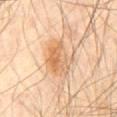Captured during whole-body skin photography for melanoma surveillance; the lesion was not biopsied. Measured at roughly 5 mm in maximum diameter. Imaged with cross-polarized lighting. A male patient aged 68 to 72. Located on the abdomen. A close-up tile cropped from a whole-body skin photograph, about 15 mm across.